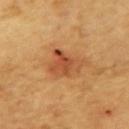follow-up: imaged on a skin check; not biopsied | patient: male, aged approximately 65 | body site: the left upper arm | lighting: cross-polarized illumination | automated metrics: an area of roughly 9 mm², an outline eccentricity of about 0.55 (0 = round, 1 = elongated), and two-axis asymmetry of about 0.4; a lesion color around L≈51 a*≈27 b*≈40 in CIELAB and a normalized border contrast of about 7.5 | image source: ~15 mm crop, total-body skin-cancer survey | lesion diameter: about 4 mm.